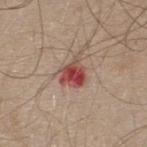Part of a total-body skin-imaging series; this lesion was reviewed on a skin check and was not flagged for biopsy. The patient is a male roughly 30 years of age. Measured at roughly 3.5 mm in maximum diameter. Imaged with white-light lighting. This image is a 15 mm lesion crop taken from a total-body photograph. The lesion is on the upper back. An algorithmic analysis of the crop reported a border-irregularity index near 4/10 and a color-variation rating of about 7/10.